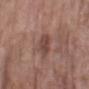patient = female, in their 70s; location = the left forearm; image = 15 mm crop, total-body photography.